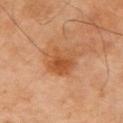Part of a total-body skin-imaging series; this lesion was reviewed on a skin check and was not flagged for biopsy.
This is a cross-polarized tile.
A 15 mm close-up tile from a total-body photography series done for melanoma screening.
Located on the right upper arm.
The lesion's longest dimension is about 4.5 mm.
A male subject, in their mid- to late 60s.